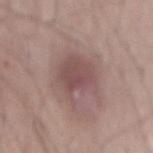Q: Was this lesion biopsied?
A: catalogued during a skin exam; not biopsied
Q: Automated lesion metrics?
A: a shape eccentricity near 0.7 and two-axis asymmetry of about 0.3; about 10 CIELAB-L* units darker than the surrounding skin and a normalized lesion–skin contrast near 7; a border-irregularity index near 4/10, a within-lesion color-variation index near 3.5/10, and radial color variation of about 1; an automated nevus-likeness rating near 20 out of 100 and a lesion-detection confidence of about 100/100
Q: What is the anatomic site?
A: the mid back
Q: What are the patient's age and sex?
A: male, aged 48–52
Q: How was the tile lit?
A: white-light illumination
Q: How was this image acquired?
A: ~15 mm tile from a whole-body skin photo